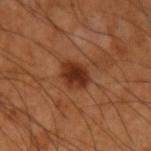The lesion was photographed on a routine skin check and not biopsied; there is no pathology result. The lesion is located on the left upper arm. Cropped from a whole-body photographic skin survey; the tile spans about 15 mm. The total-body-photography lesion software estimated an automated nevus-likeness rating near 80 out of 100. The recorded lesion diameter is about 3.5 mm. The tile uses cross-polarized illumination. A male patient aged 63 to 67.